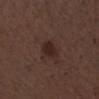| feature | finding |
|---|---|
| biopsy status | no biopsy performed (imaged during a skin exam) |
| patient | male, aged 48–52 |
| acquisition | ~15 mm tile from a whole-body skin photo |
| size | ~3 mm (longest diameter) |